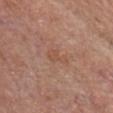<case>
<biopsy_status>not biopsied; imaged during a skin examination</biopsy_status>
<lesion_size>
  <long_diameter_mm_approx>3.0</long_diameter_mm_approx>
</lesion_size>
<automated_metrics>
  <eccentricity>0.85</eccentricity>
  <shape_asymmetry>0.5</shape_asymmetry>
  <nevus_likeness_0_100>0</nevus_likeness_0_100>
  <lesion_detection_confidence_0_100>100</lesion_detection_confidence_0_100>
</automated_metrics>
<lighting>white-light</lighting>
<site>head or neck</site>
<patient>
  <sex>male</sex>
  <age_approx>85+</age_approx>
</patient>
<image>
  <source>total-body photography crop</source>
  <field_of_view_mm>15</field_of_view_mm>
</image>
</case>